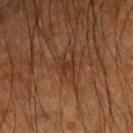Context:
The total-body-photography lesion software estimated a lesion area of about 3.5 mm². The analysis additionally found an average lesion color of about L≈30 a*≈20 b*≈29 (CIELAB) and a normalized border contrast of about 6. The software also gave a border-irregularity rating of about 4.5/10, a color-variation rating of about 2/10, and radial color variation of about 0.5. On the right forearm. The patient is a male about 60 years old. Measured at roughly 2.5 mm in maximum diameter. A 15 mm close-up tile from a total-body photography series done for melanoma screening. Imaged with cross-polarized lighting.